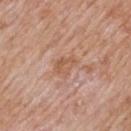The lesion was tiled from a total-body skin photograph and was not biopsied. An algorithmic analysis of the crop reported a classifier nevus-likeness of about 0/100 and a lesion-detection confidence of about 100/100. Cropped from a total-body skin-imaging series; the visible field is about 15 mm. The lesion is on the mid back. A male subject, in their 60s. Measured at roughly 3 mm in maximum diameter. Captured under white-light illumination.